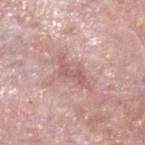- follow-up · no biopsy performed (imaged during a skin exam)
- image source · total-body-photography crop, ~15 mm field of view
- diameter · ~4.5 mm (longest diameter)
- illumination · white-light illumination
- subject · male, in their mid- to late 70s
- site · the head or neck